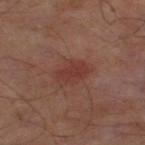Q: Is there a histopathology result?
A: imaged on a skin check; not biopsied
Q: What are the patient's age and sex?
A: male, roughly 65 years of age
Q: What did automated image analysis measure?
A: a footprint of about 7 mm², an eccentricity of roughly 0.7, and a shape-asymmetry score of about 0.25 (0 = symmetric); border irregularity of about 2.5 on a 0–10 scale, internal color variation of about 2 on a 0–10 scale, and peripheral color asymmetry of about 0.5
Q: What is the imaging modality?
A: ~15 mm crop, total-body skin-cancer survey
Q: Illumination type?
A: cross-polarized illumination
Q: How large is the lesion?
A: ~3.5 mm (longest diameter)
Q: What is the anatomic site?
A: the leg